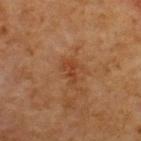Captured during whole-body skin photography for melanoma surveillance; the lesion was not biopsied. Captured under cross-polarized illumination. A male subject aged 58 to 62. The lesion is located on the upper back. The lesion-visualizer software estimated an area of roughly 4 mm² and a shape-asymmetry score of about 0.45 (0 = symmetric). It also reported a border-irregularity index near 4.5/10, a within-lesion color-variation index near 1.5/10, and a peripheral color-asymmetry measure near 0.5. It also reported an automated nevus-likeness rating near 0 out of 100. Cropped from a whole-body photographic skin survey; the tile spans about 15 mm. Measured at roughly 3 mm in maximum diameter.